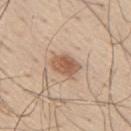Image and clinical context: Approximately 3 mm at its widest. From the right upper arm. A 15 mm close-up tile from a total-body photography series done for melanoma screening. A male subject, aged around 55.